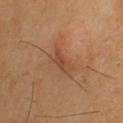biopsy_status: not biopsied; imaged during a skin examination
site: upper back
automated_metrics:
  cielab_L: 45
  cielab_a: 20
  cielab_b: 31
  vs_skin_contrast_norm: 5.0
  border_irregularity_0_10: 4.5
  color_variation_0_10: 4.0
  nevus_likeness_0_100: 5
  lesion_detection_confidence_0_100: 100
image:
  source: total-body photography crop
  field_of_view_mm: 15
lighting: cross-polarized
patient:
  sex: male
  age_approx: 70